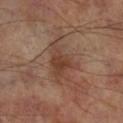<tbp_lesion>
<biopsy_status>not biopsied; imaged during a skin examination</biopsy_status>
<lesion_size>
  <long_diameter_mm_approx>5.5</long_diameter_mm_approx>
</lesion_size>
<image>
  <source>total-body photography crop</source>
  <field_of_view_mm>15</field_of_view_mm>
</image>
<site>right lower leg</site>
<patient>
  <sex>male</sex>
  <age_approx>70</age_approx>
</patient>
<lighting>cross-polarized</lighting>
</tbp_lesion>